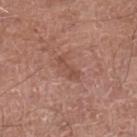patient:
  sex: male
  age_approx: 65
image:
  source: total-body photography crop
  field_of_view_mm: 15
lesion_size:
  long_diameter_mm_approx: 3.5
lighting: white-light
site: left lower leg